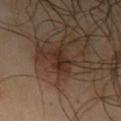  biopsy_status: not biopsied; imaged during a skin examination
  site: left upper arm
  lighting: cross-polarized
  patient:
    sex: male
    age_approx: 65
  image:
    source: total-body photography crop
    field_of_view_mm: 15
  lesion_size:
    long_diameter_mm_approx: 3.0